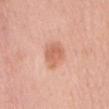size: ~3.5 mm (longest diameter) | lighting: white-light | subject: male, aged around 70 | image source: ~15 mm tile from a whole-body skin photo | anatomic site: the mid back | automated metrics: a lesion color around L≈64 a*≈25 b*≈33 in CIELAB, about 10 CIELAB-L* units darker than the surrounding skin, and a lesion-to-skin contrast of about 6.5 (normalized; higher = more distinct); a border-irregularity rating of about 2.5/10, a color-variation rating of about 2.5/10, and radial color variation of about 1.